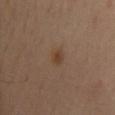biopsy status: catalogued during a skin exam; not biopsied | location: the mid back | acquisition: ~15 mm tile from a whole-body skin photo | subject: male, in their mid- to late 50s.